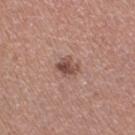Q: Lesion location?
A: the right thigh
Q: Automated lesion metrics?
A: an eccentricity of roughly 0.45 and two-axis asymmetry of about 0.15
Q: What are the patient's age and sex?
A: female, aged 48 to 52
Q: What kind of image is this?
A: ~15 mm tile from a whole-body skin photo